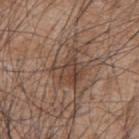Captured under white-light illumination. A region of skin cropped from a whole-body photographic capture, roughly 15 mm wide. A male patient aged around 45. The lesion is located on the upper back.